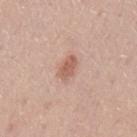Part of a total-body skin-imaging series; this lesion was reviewed on a skin check and was not flagged for biopsy. The lesion is on the left upper arm. Imaged with white-light lighting. An algorithmic analysis of the crop reported an average lesion color of about L≈59 a*≈22 b*≈28 (CIELAB) and roughly 11 lightness units darker than nearby skin. The software also gave peripheral color asymmetry of about 0.5. The software also gave an automated nevus-likeness rating near 65 out of 100. A male subject, aged 43 to 47. A roughly 15 mm field-of-view crop from a total-body skin photograph. About 3 mm across.